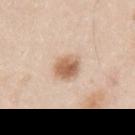Clinical impression:
The lesion was photographed on a routine skin check and not biopsied; there is no pathology result.
Background:
Cropped from a whole-body photographic skin survey; the tile spans about 15 mm. On the left upper arm. The patient is a male aged approximately 35. About 3 mm across.